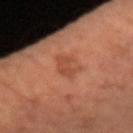| key | value |
|---|---|
| follow-up | catalogued during a skin exam; not biopsied |
| imaging modality | 15 mm crop, total-body photography |
| patient | male, roughly 50 years of age |
| automated lesion analysis | a lesion area of about 5 mm², an outline eccentricity of about 0.85 (0 = round, 1 = elongated), and two-axis asymmetry of about 0.35; lesion-presence confidence of about 100/100 |
| body site | the right forearm |
| size | about 3.5 mm |